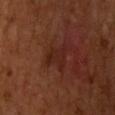| key | value |
|---|---|
| lesion size | ~3.5 mm (longest diameter) |
| illumination | cross-polarized illumination |
| body site | the upper back |
| patient | male, aged 58 to 62 |
| automated lesion analysis | an area of roughly 5 mm², a shape eccentricity near 0.7, and a shape-asymmetry score of about 0.5 (0 = symmetric); about 5 CIELAB-L* units darker than the surrounding skin and a normalized lesion–skin contrast near 5.5 |
| image source | ~15 mm crop, total-body skin-cancer survey |